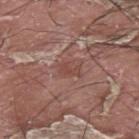| feature | finding |
|---|---|
| subject | male, roughly 40 years of age |
| location | the back |
| imaging modality | ~15 mm tile from a whole-body skin photo |
| TBP lesion metrics | a lesion area of about 4 mm², an eccentricity of roughly 0.8, and a shape-asymmetry score of about 0.3 (0 = symmetric); about 7 CIELAB-L* units darker than the surrounding skin and a normalized border contrast of about 5.5; a peripheral color-asymmetry measure near 0; a nevus-likeness score of about 0/100 |